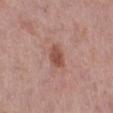notes: imaged on a skin check; not biopsied
illumination: white-light
lesion diameter: ≈3 mm
image-analysis metrics: a lesion color around L≈50 a*≈24 b*≈27 in CIELAB and a normalized border contrast of about 8; internal color variation of about 2.5 on a 0–10 scale and a peripheral color-asymmetry measure near 1; a lesion-detection confidence of about 100/100
body site: the right lower leg
imaging modality: total-body-photography crop, ~15 mm field of view
patient: female, in their 40s